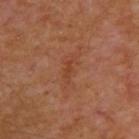The lesion was tiled from a total-body skin photograph and was not biopsied. The recorded lesion diameter is about 3 mm. A 15 mm close-up extracted from a 3D total-body photography capture. The subject is a male aged 53–57. The lesion is on the upper back. This is a cross-polarized tile.